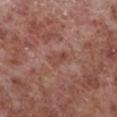Impression:
Recorded during total-body skin imaging; not selected for excision or biopsy.
Background:
A roughly 15 mm field-of-view crop from a total-body skin photograph. A male subject aged 53–57. Approximately 3 mm at its widest. This is a white-light tile. From the left lower leg.